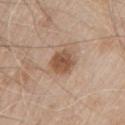- notes: total-body-photography surveillance lesion; no biopsy
- subject: male, approximately 80 years of age
- acquisition: 15 mm crop, total-body photography
- diameter: ≈3 mm
- site: the chest
- tile lighting: white-light